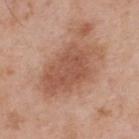follow-up = no biopsy performed (imaged during a skin exam) | body site = the upper back | subject = male, aged approximately 55 | lesion diameter = ≈8 mm | tile lighting = white-light | image source = ~15 mm tile from a whole-body skin photo.